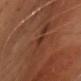biopsy status=catalogued during a skin exam; not biopsied
patient=male, approximately 65 years of age
image-analysis metrics=a lesion area of about 1.5 mm²; an automated nevus-likeness rating near 0 out of 100
illumination=cross-polarized
image=~15 mm tile from a whole-body skin photo
body site=the front of the torso